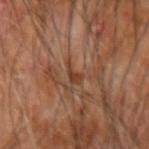Imaged during a routine full-body skin examination; the lesion was not biopsied and no histopathology is available.
A roughly 15 mm field-of-view crop from a total-body skin photograph.
An algorithmic analysis of the crop reported a lesion area of about 3 mm², a shape eccentricity near 0.85, and two-axis asymmetry of about 0.5. It also reported about 8 CIELAB-L* units darker than the surrounding skin and a normalized border contrast of about 6.5. And it measured a border-irregularity rating of about 5/10, a within-lesion color-variation index near 2.5/10, and peripheral color asymmetry of about 1.
Longest diameter approximately 2.5 mm.
The tile uses cross-polarized illumination.
The lesion is on the arm.
A male subject aged approximately 65.